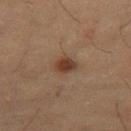notes: imaged on a skin check; not biopsied
size: ≈2.5 mm
TBP lesion metrics: a lesion area of about 4.5 mm², an eccentricity of roughly 0.6, and a shape-asymmetry score of about 0.35 (0 = symmetric); a lesion color around L≈33 a*≈16 b*≈25 in CIELAB, roughly 10 lightness units darker than nearby skin, and a lesion-to-skin contrast of about 9 (normalized; higher = more distinct); a border-irregularity rating of about 3/10, a color-variation rating of about 3/10, and radial color variation of about 1
image: 15 mm crop, total-body photography
body site: the right thigh
patient: female, approximately 55 years of age
tile lighting: cross-polarized illumination